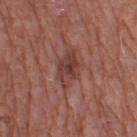No biopsy was performed on this lesion — it was imaged during a full skin examination and was not determined to be concerning. Captured under white-light illumination. Located on the right thigh. An algorithmic analysis of the crop reported an automated nevus-likeness rating near 0 out of 100 and a lesion-detection confidence of about 100/100. The patient is a male approximately 75 years of age. About 4.5 mm across. A roughly 15 mm field-of-view crop from a total-body skin photograph.